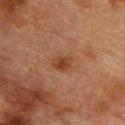workup: imaged on a skin check; not biopsied
image source: ~15 mm tile from a whole-body skin photo
patient: male, roughly 75 years of age
site: the chest
illumination: cross-polarized